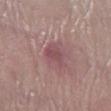{
  "biopsy_status": "not biopsied; imaged during a skin examination",
  "site": "left lower leg",
  "image": {
    "source": "total-body photography crop",
    "field_of_view_mm": 15
  },
  "automated_metrics": {
    "area_mm2_approx": 6.0,
    "lesion_detection_confidence_0_100": 100
  },
  "lesion_size": {
    "long_diameter_mm_approx": 3.5
  },
  "patient": {
    "sex": "male",
    "age_approx": 70
  }
}